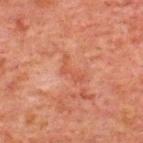Q: Was this lesion biopsied?
A: total-body-photography surveillance lesion; no biopsy
Q: Patient demographics?
A: male, approximately 60 years of age
Q: What is the anatomic site?
A: the upper back
Q: Lesion size?
A: about 3.5 mm
Q: What did automated image analysis measure?
A: a border-irregularity index near 9/10, a color-variation rating of about 0/10, and a peripheral color-asymmetry measure near 0
Q: What lighting was used for the tile?
A: cross-polarized illumination
Q: How was this image acquired?
A: ~15 mm tile from a whole-body skin photo